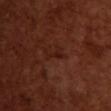biopsy status=no biopsy performed (imaged during a skin exam); illumination=cross-polarized; anatomic site=the upper back; subject=female, aged approximately 55; imaging modality=~15 mm crop, total-body skin-cancer survey.